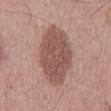  biopsy_status: not biopsied; imaged during a skin examination
  lesion_size:
    long_diameter_mm_approx: 8.5
  site: mid back
  automated_metrics:
    area_mm2_approx: 27.0
    eccentricity: 0.9
    shape_asymmetry: 0.1
    border_irregularity_0_10: 2.0
    color_variation_0_10: 3.0
    peripheral_color_asymmetry: 1.0
  image:
    source: total-body photography crop
    field_of_view_mm: 15
  patient:
    sex: male
    age_approx: 55
  lighting: white-light